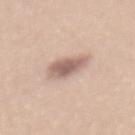workup = no biopsy performed (imaged during a skin exam)
subject = female, about 35 years old
image source = total-body-photography crop, ~15 mm field of view
body site = the mid back